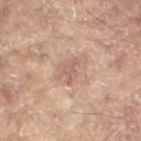This lesion was catalogued during total-body skin photography and was not selected for biopsy. Located on the left leg. The recorded lesion diameter is about 3 mm. Automated tile analysis of the lesion measured border irregularity of about 7.5 on a 0–10 scale. The patient is a female in their 80s. The tile uses cross-polarized illumination. A roughly 15 mm field-of-view crop from a total-body skin photograph.